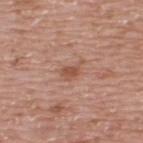Assessment:
Captured during whole-body skin photography for melanoma surveillance; the lesion was not biopsied.
Context:
Located on the upper back. Captured under white-light illumination. A roughly 15 mm field-of-view crop from a total-body skin photograph. The subject is a male aged 73 to 77.